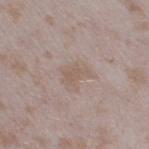<record>
<biopsy_status>not biopsied; imaged during a skin examination</biopsy_status>
<automated_metrics>
  <cielab_L>56</cielab_L>
  <cielab_a>14</cielab_a>
  <cielab_b>24</cielab_b>
  <vs_skin_darker_L>6.0</vs_skin_darker_L>
  <vs_skin_contrast_norm>5.5</vs_skin_contrast_norm>
  <nevus_likeness_0_100>0</nevus_likeness_0_100>
  <lesion_detection_confidence_0_100>100</lesion_detection_confidence_0_100>
</automated_metrics>
<image>
  <source>total-body photography crop</source>
  <field_of_view_mm>15</field_of_view_mm>
</image>
<patient>
  <sex>female</sex>
  <age_approx>25</age_approx>
</patient>
<site>right thigh</site>
<lighting>white-light</lighting>
</record>